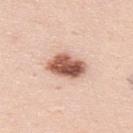Case summary:
– illumination · white-light illumination
– patient · male, aged around 35
– anatomic site · the upper back
– automated lesion analysis · an average lesion color of about L≈58 a*≈23 b*≈29 (CIELAB), about 20 CIELAB-L* units darker than the surrounding skin, and a normalized lesion–skin contrast near 12; an automated nevus-likeness rating near 95 out of 100 and a detector confidence of about 100 out of 100 that the crop contains a lesion
– image source · ~15 mm tile from a whole-body skin photo